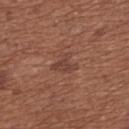Q: Is there a histopathology result?
A: catalogued during a skin exam; not biopsied
Q: How large is the lesion?
A: ~3.5 mm (longest diameter)
Q: Patient demographics?
A: female, aged around 65
Q: How was this image acquired?
A: ~15 mm crop, total-body skin-cancer survey
Q: Illumination type?
A: white-light
Q: What is the anatomic site?
A: the upper back